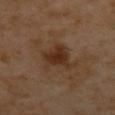Assessment: No biopsy was performed on this lesion — it was imaged during a full skin examination and was not determined to be concerning. Background: Automated image analysis of the tile measured an outline eccentricity of about 0.7 (0 = round, 1 = elongated) and a symmetry-axis asymmetry near 0.3. And it measured a classifier nevus-likeness of about 80/100 and a lesion-detection confidence of about 100/100. A 15 mm crop from a total-body photograph taken for skin-cancer surveillance. Located on the mid back. The tile uses cross-polarized illumination. A female subject, in their mid-50s.